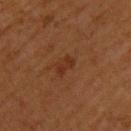The tile uses cross-polarized illumination.
Cropped from a whole-body photographic skin survey; the tile spans about 15 mm.
Approximately 2.5 mm at its widest.
On the upper back.
A male subject in their mid- to late 50s.